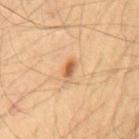Part of a total-body skin-imaging series; this lesion was reviewed on a skin check and was not flagged for biopsy. About 2.5 mm across. The patient is a male aged 53 to 57. The lesion is on the mid back. A 15 mm crop from a total-body photograph taken for skin-cancer surveillance.